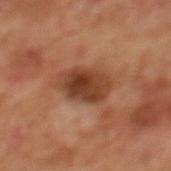Case summary:
- workup — catalogued during a skin exam; not biopsied
- diameter — ~5 mm (longest diameter)
- patient — male, roughly 70 years of age
- automated lesion analysis — a footprint of about 13 mm², a shape eccentricity near 0.8, and a symmetry-axis asymmetry near 0.15; internal color variation of about 5.5 on a 0–10 scale and peripheral color asymmetry of about 1.5; a lesion-detection confidence of about 100/100
- imaging modality — 15 mm crop, total-body photography
- body site — the mid back
- tile lighting — cross-polarized